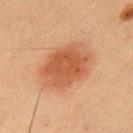The lesion was photographed on a routine skin check and not biopsied; there is no pathology result. The lesion is on the left upper arm. A 15 mm close-up extracted from a 3D total-body photography capture. Longest diameter approximately 6.5 mm. The tile uses cross-polarized illumination. The subject is a male in their 60s.